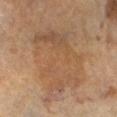Recorded during total-body skin imaging; not selected for excision or biopsy.
On the right lower leg.
A female patient, aged 68 to 72.
A lesion tile, about 15 mm wide, cut from a 3D total-body photograph.